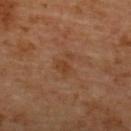follow-up — total-body-photography surveillance lesion; no biopsy
TBP lesion metrics — a footprint of about 5.5 mm², a shape eccentricity near 0.65, and two-axis asymmetry of about 0.5; a mean CIELAB color near L≈42 a*≈21 b*≈33 and roughly 5 lightness units darker than nearby skin; lesion-presence confidence of about 100/100
lighting — cross-polarized illumination
location — the upper back
patient — female, about 55 years old
acquisition — ~15 mm crop, total-body skin-cancer survey